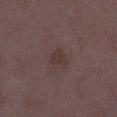Notes:
• biopsy status · catalogued during a skin exam; not biopsied
• patient · female, aged 33–37
• acquisition · total-body-photography crop, ~15 mm field of view
• site · the leg
• automated lesion analysis · a classifier nevus-likeness of about 0/100 and a lesion-detection confidence of about 100/100
• illumination · white-light